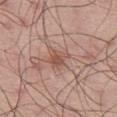The lesion was tiled from a total-body skin photograph and was not biopsied. Captured under white-light illumination. From the back. A male patient, aged approximately 45. Automated image analysis of the tile measured an area of roughly 4.5 mm² and a shape-asymmetry score of about 0.25 (0 = symmetric). The analysis additionally found a border-irregularity index near 3/10, a within-lesion color-variation index near 2.5/10, and peripheral color asymmetry of about 0.5. A 15 mm crop from a total-body photograph taken for skin-cancer surveillance.